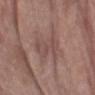Impression:
Captured during whole-body skin photography for melanoma surveillance; the lesion was not biopsied.
Context:
The lesion is located on the left forearm. A lesion tile, about 15 mm wide, cut from a 3D total-body photograph. The patient is a female aged 68–72. The total-body-photography lesion software estimated an eccentricity of roughly 0.8. The analysis additionally found a color-variation rating of about 2.5/10 and a peripheral color-asymmetry measure near 1.